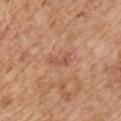Q: What is the imaging modality?
A: ~15 mm tile from a whole-body skin photo
Q: What is the anatomic site?
A: the right upper arm
Q: Who is the patient?
A: male, approximately 65 years of age